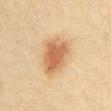Case summary:
* notes: no biopsy performed (imaged during a skin exam)
* subject: male, roughly 40 years of age
* automated metrics: an average lesion color of about L≈56 a*≈20 b*≈35 (CIELAB), about 12 CIELAB-L* units darker than the surrounding skin, and a normalized border contrast of about 8.5; a border-irregularity rating of about 2.5/10, a within-lesion color-variation index near 3.5/10, and radial color variation of about 1
* tile lighting: cross-polarized illumination
* image source: total-body-photography crop, ~15 mm field of view
* size: ≈5 mm
* anatomic site: the arm